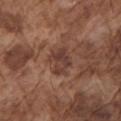Assessment: Captured during whole-body skin photography for melanoma surveillance; the lesion was not biopsied. Clinical summary: The lesion is on the left upper arm. This is a white-light tile. Longest diameter approximately 3.5 mm. A 15 mm crop from a total-body photograph taken for skin-cancer surveillance. A male subject, roughly 75 years of age.